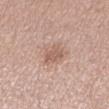The lesion was tiled from a total-body skin photograph and was not biopsied. The tile uses white-light illumination. The patient is a female aged 53–57. Cropped from a total-body skin-imaging series; the visible field is about 15 mm. The lesion is located on the left lower leg.